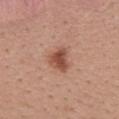Notes:
- workup — catalogued during a skin exam; not biopsied
- subject — female, aged 23 to 27
- site — the upper back
- illumination — white-light
- size — ≈3.5 mm
- image — 15 mm crop, total-body photography